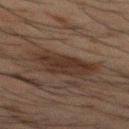Impression: Recorded during total-body skin imaging; not selected for excision or biopsy. Clinical summary: Imaged with cross-polarized lighting. A 15 mm crop from a total-body photograph taken for skin-cancer surveillance. The lesion is located on the mid back. About 6 mm across. The patient is a male in their 50s.